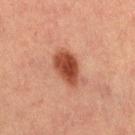Part of a total-body skin-imaging series; this lesion was reviewed on a skin check and was not flagged for biopsy. Located on the right thigh. About 4.5 mm across. A female patient aged approximately 40. A roughly 15 mm field-of-view crop from a total-body skin photograph.